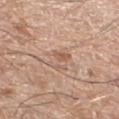Assessment:
The lesion was photographed on a routine skin check and not biopsied; there is no pathology result.
Acquisition and patient details:
A 15 mm close-up extracted from a 3D total-body photography capture. Measured at roughly 3.5 mm in maximum diameter. Located on the left thigh. The patient is a male approximately 70 years of age. This is a white-light tile.